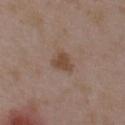Case summary:
• workup · no biopsy performed (imaged during a skin exam)
• anatomic site · the chest
• patient · female, roughly 35 years of age
• image source · 15 mm crop, total-body photography
• lesion diameter · ~2.5 mm (longest diameter)